The lesion is on the right thigh · cropped from a total-body skin-imaging series; the visible field is about 15 mm · a female patient, roughly 45 years of age:
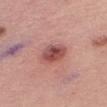  lighting: white-light
  lesion_size:
    long_diameter_mm_approx: 3.5
  diagnosis:
    histopathology: intradermal melanocytic nevus
    malignancy: benign
    taxonomic_path:
      - Benign
      - Benign melanocytic proliferations
      - Nevus
      - Nevus, NOS, Dermal